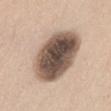biopsy status: no biopsy performed (imaged during a skin exam) | site: the left thigh | lighting: white-light illumination | lesion size: ~8 mm (longest diameter) | imaging modality: ~15 mm tile from a whole-body skin photo | subject: male, approximately 60 years of age.